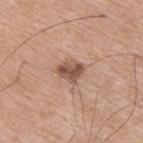biopsy status: imaged on a skin check; not biopsied | site: the upper back | diameter: ≈3 mm | image: total-body-photography crop, ~15 mm field of view | tile lighting: white-light | subject: male, roughly 75 years of age.